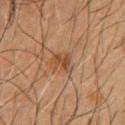Assessment:
Imaged during a routine full-body skin examination; the lesion was not biopsied and no histopathology is available.
Acquisition and patient details:
A male subject, aged around 65. Located on the chest. Cropped from a whole-body photographic skin survey; the tile spans about 15 mm.